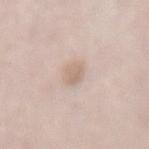A female subject about 30 years old. On the mid back. Cropped from a whole-body photographic skin survey; the tile spans about 15 mm.